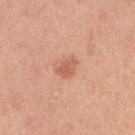This lesion was catalogued during total-body skin photography and was not selected for biopsy.
Captured under white-light illumination.
Located on the right upper arm.
A region of skin cropped from a whole-body photographic capture, roughly 15 mm wide.
The subject is a female aged around 45.